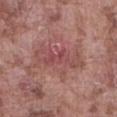workup = no biopsy performed (imaged during a skin exam) | subject = male, aged 73–77 | acquisition = ~15 mm tile from a whole-body skin photo | anatomic site = the abdomen | lighting = white-light.